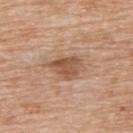follow-up — imaged on a skin check; not biopsied | body site — the upper back | image source — ~15 mm crop, total-body skin-cancer survey | subject — female, aged 73–77.